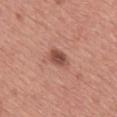Part of a total-body skin-imaging series; this lesion was reviewed on a skin check and was not flagged for biopsy.